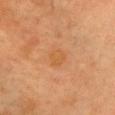Assessment:
Imaged during a routine full-body skin examination; the lesion was not biopsied and no histopathology is available.
Image and clinical context:
A female patient approximately 60 years of age. From the head or neck. Automated image analysis of the tile measured a lesion–skin lightness drop of about 5 and a lesion-to-skin contrast of about 5.5 (normalized; higher = more distinct). The software also gave a nevus-likeness score of about 0/100 and lesion-presence confidence of about 100/100. A region of skin cropped from a whole-body photographic capture, roughly 15 mm wide.